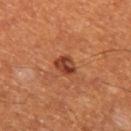Q: Was a biopsy performed?
A: imaged on a skin check; not biopsied